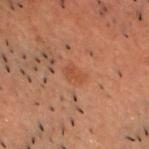Q: Was this lesion biopsied?
A: imaged on a skin check; not biopsied
Q: How was this image acquired?
A: ~15 mm crop, total-body skin-cancer survey
Q: Who is the patient?
A: male, aged 48 to 52
Q: What is the anatomic site?
A: the chest